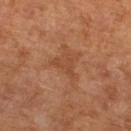Assessment:
The lesion was photographed on a routine skin check and not biopsied; there is no pathology result.
Acquisition and patient details:
The patient is a female in their mid-60s. The lesion is on the left lower leg. A close-up tile cropped from a whole-body skin photograph, about 15 mm across. Approximately 3.5 mm at its widest. This is a cross-polarized tile.